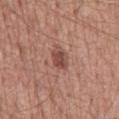Captured during whole-body skin photography for melanoma surveillance; the lesion was not biopsied. This is a white-light tile. Measured at roughly 2.5 mm in maximum diameter. Cropped from a total-body skin-imaging series; the visible field is about 15 mm. The subject is a male in their mid- to late 50s. On the mid back. An algorithmic analysis of the crop reported a lesion–skin lightness drop of about 11 and a lesion-to-skin contrast of about 8 (normalized; higher = more distinct). And it measured a nevus-likeness score of about 60/100 and lesion-presence confidence of about 100/100.